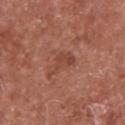notes=no biopsy performed (imaged during a skin exam); site=the front of the torso; patient=male, approximately 65 years of age; lesion size=about 4 mm; lighting=white-light illumination; image=total-body-photography crop, ~15 mm field of view.